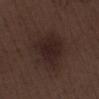<lesion>
<biopsy_status>not biopsied; imaged during a skin examination</biopsy_status>
<patient>
  <sex>male</sex>
  <age_approx>70</age_approx>
</patient>
<site>right lower leg</site>
<lighting>white-light</lighting>
<image>
  <source>total-body photography crop</source>
  <field_of_view_mm>15</field_of_view_mm>
</image>
<automated_metrics>
  <color_variation_0_10>2.5</color_variation_0_10>
  <peripheral_color_asymmetry>0.5</peripheral_color_asymmetry>
  <nevus_likeness_0_100>35</nevus_likeness_0_100>
  <lesion_detection_confidence_0_100>100</lesion_detection_confidence_0_100>
</automated_metrics>
</lesion>